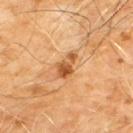workup: total-body-photography surveillance lesion; no biopsy | subject: male, about 60 years old | site: the chest | diameter: about 3.5 mm | illumination: cross-polarized illumination | image: 15 mm crop, total-body photography.